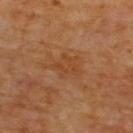A region of skin cropped from a whole-body photographic capture, roughly 15 mm wide.
The subject is a male aged around 60.
An algorithmic analysis of the crop reported a mean CIELAB color near L≈43 a*≈22 b*≈35, roughly 5 lightness units darker than nearby skin, and a lesion-to-skin contrast of about 5 (normalized; higher = more distinct). The software also gave border irregularity of about 5.5 on a 0–10 scale, internal color variation of about 2 on a 0–10 scale, and a peripheral color-asymmetry measure near 1.
Located on the upper back.
The recorded lesion diameter is about 4.5 mm.